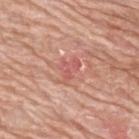Impression:
The lesion was tiled from a total-body skin photograph and was not biopsied.
Context:
Measured at roughly 3 mm in maximum diameter. The total-body-photography lesion software estimated two-axis asymmetry of about 0.45. And it measured a mean CIELAB color near L≈58 a*≈29 b*≈27, about 7 CIELAB-L* units darker than the surrounding skin, and a normalized lesion–skin contrast near 5. The analysis additionally found a border-irregularity rating of about 5/10, internal color variation of about 2.5 on a 0–10 scale, and peripheral color asymmetry of about 1. The subject is a male aged approximately 80. Cropped from a total-body skin-imaging series; the visible field is about 15 mm. Captured under white-light illumination. The lesion is on the upper back.